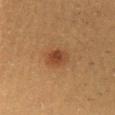Impression:
The lesion was tiled from a total-body skin photograph and was not biopsied.
Acquisition and patient details:
The lesion is on the chest. Approximately 3 mm at its widest. A female patient aged around 40. Cropped from a total-body skin-imaging series; the visible field is about 15 mm. The tile uses cross-polarized illumination.